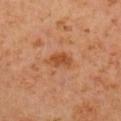image-analysis metrics: an eccentricity of roughly 0.8 and a symmetry-axis asymmetry near 0.25; a lesion–skin lightness drop of about 8 and a lesion-to-skin contrast of about 7 (normalized; higher = more distinct); a classifier nevus-likeness of about 45/100 and a detector confidence of about 100 out of 100 that the crop contains a lesion | lesion size: ~3.5 mm (longest diameter) | acquisition: ~15 mm crop, total-body skin-cancer survey | illumination: cross-polarized illumination | subject: male, aged 58–62 | anatomic site: the right upper arm.